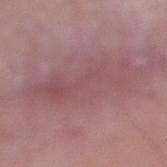Notes:
• follow-up — total-body-photography surveillance lesion; no biopsy
• imaging modality — ~15 mm crop, total-body skin-cancer survey
• lesion diameter — about 10 mm
• subject — male, roughly 85 years of age or older
• tile lighting — white-light
• site — the right lower leg
• TBP lesion metrics — border irregularity of about 5.5 on a 0–10 scale and a color-variation rating of about 3.5/10; a classifier nevus-likeness of about 0/100 and lesion-presence confidence of about 60/100